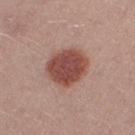| field | value |
|---|---|
| notes | catalogued during a skin exam; not biopsied |
| anatomic site | the right thigh |
| image | ~15 mm crop, total-body skin-cancer survey |
| subject | female, aged around 25 |